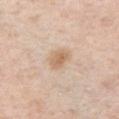Q: Was a biopsy performed?
A: catalogued during a skin exam; not biopsied
Q: Who is the patient?
A: male, roughly 50 years of age
Q: What kind of image is this?
A: ~15 mm tile from a whole-body skin photo
Q: What is the anatomic site?
A: the chest
Q: Lesion size?
A: ~3 mm (longest diameter)